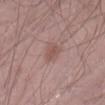The lesion was photographed on a routine skin check and not biopsied; there is no pathology result. Automated image analysis of the tile measured an area of roughly 4.5 mm², an outline eccentricity of about 0.65 (0 = round, 1 = elongated), and a shape-asymmetry score of about 0.35 (0 = symmetric). And it measured a mean CIELAB color near L≈52 a*≈20 b*≈22 and a normalized border contrast of about 5.5. The analysis additionally found a border-irregularity rating of about 3/10, a color-variation rating of about 2.5/10, and peripheral color asymmetry of about 1. Imaged with white-light lighting. Cropped from a total-body skin-imaging series; the visible field is about 15 mm. The lesion is on the right thigh. About 3 mm across. The subject is a male aged approximately 55.